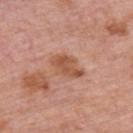{
  "biopsy_status": "not biopsied; imaged during a skin examination",
  "patient": {
    "sex": "male",
    "age_approx": 75
  },
  "automated_metrics": {
    "area_mm2_approx": 8.0,
    "eccentricity": 0.8,
    "cielab_L": 54,
    "cielab_a": 24,
    "cielab_b": 32,
    "vs_skin_darker_L": 10.0,
    "vs_skin_contrast_norm": 7.5
  },
  "site": "upper back",
  "lighting": "white-light",
  "image": {
    "source": "total-body photography crop",
    "field_of_view_mm": 15
  }
}